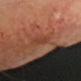Part of a total-body skin-imaging series; this lesion was reviewed on a skin check and was not flagged for biopsy. From the head or neck. Imaged with cross-polarized lighting. A female subject roughly 75 years of age. A 15 mm close-up extracted from a 3D total-body photography capture.